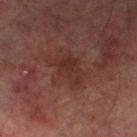The subject is a male about 75 years old. Located on the left lower leg. A lesion tile, about 15 mm wide, cut from a 3D total-body photograph.